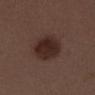The lesion was photographed on a routine skin check and not biopsied; there is no pathology result. Imaged with white-light lighting. The lesion is on the back. A region of skin cropped from a whole-body photographic capture, roughly 15 mm wide. An algorithmic analysis of the crop reported a normalized border contrast of about 10. The analysis additionally found a border-irregularity index near 1/10, a color-variation rating of about 3.5/10, and radial color variation of about 1. And it measured lesion-presence confidence of about 100/100. A male patient, in their 30s. Longest diameter approximately 5 mm.